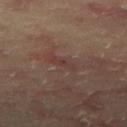follow-up = catalogued during a skin exam; not biopsied
lesion diameter = ~3.5 mm (longest diameter)
tile lighting = cross-polarized
patient = male, in their mid- to late 60s
acquisition = 15 mm crop, total-body photography
anatomic site = the right thigh